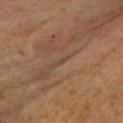{"biopsy_status": "not biopsied; imaged during a skin examination", "automated_metrics": {"eccentricity": 0.95, "shape_asymmetry": 0.45, "vs_skin_darker_L": 4.0, "vs_skin_contrast_norm": 3.5}, "lighting": "cross-polarized", "site": "right upper arm", "lesion_size": {"long_diameter_mm_approx": 2.0}, "patient": {"sex": "female", "age_approx": 40}, "image": {"source": "total-body photography crop", "field_of_view_mm": 15}}